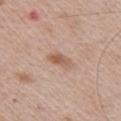<lesion>
  <biopsy_status>not biopsied; imaged during a skin examination</biopsy_status>
  <lighting>white-light</lighting>
  <site>chest</site>
  <patient>
    <sex>male</sex>
    <age_approx>65</age_approx>
  </patient>
  <image>
    <source>total-body photography crop</source>
    <field_of_view_mm>15</field_of_view_mm>
  </image>
</lesion>